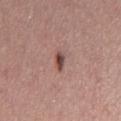automated metrics — a border-irregularity rating of about 3/10, a within-lesion color-variation index near 6/10, and peripheral color asymmetry of about 2
image — 15 mm crop, total-body photography
body site — the chest
tile lighting — white-light illumination
patient — male, aged 38–42